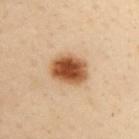Findings:
• notes: total-body-photography surveillance lesion; no biopsy
• site: the chest
• acquisition: total-body-photography crop, ~15 mm field of view
• subject: female, aged 48 to 52